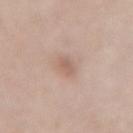No biopsy was performed on this lesion — it was imaged during a full skin examination and was not determined to be concerning. This image is a 15 mm lesion crop taken from a total-body photograph. On the back. The total-body-photography lesion software estimated a footprint of about 4 mm² and a shape eccentricity near 0.8. It also reported a border-irregularity index near 1.5/10, internal color variation of about 1.5 on a 0–10 scale, and radial color variation of about 0.5. A female subject aged 58 to 62. The tile uses white-light illumination.